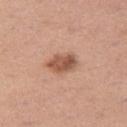Clinical impression: No biopsy was performed on this lesion — it was imaged during a full skin examination and was not determined to be concerning. Background: Automated tile analysis of the lesion measured an area of roughly 7.5 mm², an outline eccentricity of about 0.75 (0 = round, 1 = elongated), and a symmetry-axis asymmetry near 0.2. It also reported a within-lesion color-variation index near 4.5/10. Approximately 4 mm at its widest. Cropped from a whole-body photographic skin survey; the tile spans about 15 mm. From the leg. Imaged with white-light lighting. A female patient, aged approximately 30.